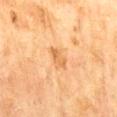Recorded during total-body skin imaging; not selected for excision or biopsy.
A close-up tile cropped from a whole-body skin photograph, about 15 mm across.
The lesion is on the mid back.
The subject is a male in their 70s.
This is a cross-polarized tile.